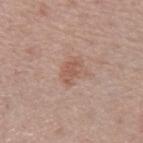Clinical summary: Captured under white-light illumination. An algorithmic analysis of the crop reported a lesion color around L≈55 a*≈21 b*≈27 in CIELAB, a lesion–skin lightness drop of about 8, and a normalized lesion–skin contrast near 6. The software also gave a detector confidence of about 100 out of 100 that the crop contains a lesion. A region of skin cropped from a whole-body photographic capture, roughly 15 mm wide. A male subject, approximately 45 years of age. The lesion is on the left forearm. Measured at roughly 3 mm in maximum diameter.